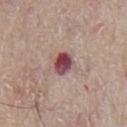Captured during whole-body skin photography for melanoma surveillance; the lesion was not biopsied. Measured at roughly 3 mm in maximum diameter. Captured under white-light illumination. On the chest. A close-up tile cropped from a whole-body skin photograph, about 15 mm across. Automated tile analysis of the lesion measured a footprint of about 6 mm², an outline eccentricity of about 0.45 (0 = round, 1 = elongated), and a symmetry-axis asymmetry near 0.15. The software also gave a mean CIELAB color near L≈46 a*≈26 b*≈17, about 17 CIELAB-L* units darker than the surrounding skin, and a normalized border contrast of about 12.5. The analysis additionally found an automated nevus-likeness rating near 0 out of 100 and lesion-presence confidence of about 100/100. A male subject, approximately 65 years of age.